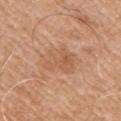Clinical impression:
The lesion was tiled from a total-body skin photograph and was not biopsied.
Clinical summary:
From the arm. A male patient, approximately 75 years of age. The total-body-photography lesion software estimated an area of roughly 12 mm², a shape eccentricity near 0.45, and a shape-asymmetry score of about 0.25 (0 = symmetric). And it measured a lesion color around L≈58 a*≈22 b*≈34 in CIELAB and roughly 6 lightness units darker than nearby skin. It also reported a border-irregularity rating of about 3.5/10, internal color variation of about 3.5 on a 0–10 scale, and peripheral color asymmetry of about 1. It also reported a classifier nevus-likeness of about 0/100 and a detector confidence of about 100 out of 100 that the crop contains a lesion. A 15 mm close-up tile from a total-body photography series done for melanoma screening. The tile uses white-light illumination.